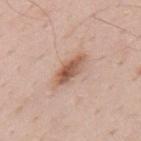Impression: Part of a total-body skin-imaging series; this lesion was reviewed on a skin check and was not flagged for biopsy. Acquisition and patient details: From the mid back. A close-up tile cropped from a whole-body skin photograph, about 15 mm across. The subject is a male aged 68 to 72.